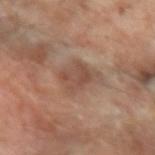| feature | finding |
|---|---|
| follow-up | imaged on a skin check; not biopsied |
| tile lighting | cross-polarized |
| location | the left forearm |
| image-analysis metrics | a lesion–skin lightness drop of about 8; an automated nevus-likeness rating near 0 out of 100 and lesion-presence confidence of about 95/100 |
| lesion size | about 4 mm |
| imaging modality | ~15 mm crop, total-body skin-cancer survey |
| subject | female, in their 70s |